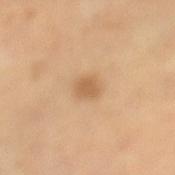Notes:
• follow-up — imaged on a skin check; not biopsied
• diameter — about 2.5 mm
• subject — female, aged 48–52
• body site — the left lower leg
• illumination — cross-polarized
• image — 15 mm crop, total-body photography